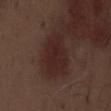Impression:
Imaged during a routine full-body skin examination; the lesion was not biopsied and no histopathology is available.